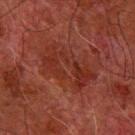No biopsy was performed on this lesion — it was imaged during a full skin examination and was not determined to be concerning.
This is a cross-polarized tile.
The lesion is located on the right forearm.
A male patient, aged 78–82.
Approximately 6.5 mm at its widest.
A 15 mm close-up extracted from a 3D total-body photography capture.